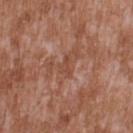Imaged during a routine full-body skin examination; the lesion was not biopsied and no histopathology is available.
On the upper back.
Approximately 2.5 mm at its widest.
A male patient, approximately 45 years of age.
A lesion tile, about 15 mm wide, cut from a 3D total-body photograph.